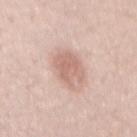Acquisition and patient details: From the mid back. Automated tile analysis of the lesion measured a lesion color around L≈65 a*≈20 b*≈25 in CIELAB, a lesion–skin lightness drop of about 11, and a normalized border contrast of about 6.5. The software also gave border irregularity of about 3.5 on a 0–10 scale and a within-lesion color-variation index near 2.5/10. The analysis additionally found an automated nevus-likeness rating near 40 out of 100. A region of skin cropped from a whole-body photographic capture, roughly 15 mm wide. Approximately 4.5 mm at its widest. A male subject, in their mid- to late 20s. Imaged with white-light lighting.